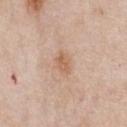Q: Was this lesion biopsied?
A: no biopsy performed (imaged during a skin exam)
Q: Lesion size?
A: about 3 mm
Q: Illumination type?
A: white-light
Q: What is the imaging modality?
A: 15 mm crop, total-body photography
Q: Patient demographics?
A: male, roughly 60 years of age
Q: What did automated image analysis measure?
A: an area of roughly 4.5 mm² and an outline eccentricity of about 0.75 (0 = round, 1 = elongated); peripheral color asymmetry of about 0.5; a classifier nevus-likeness of about 25/100 and lesion-presence confidence of about 100/100
Q: What is the anatomic site?
A: the chest